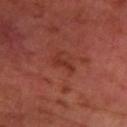  biopsy_status: not biopsied; imaged during a skin examination
  lighting: cross-polarized
  site: left forearm
  image:
    source: total-body photography crop
    field_of_view_mm: 15
  patient:
    age_approx: 65